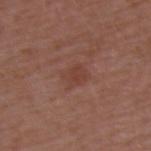Captured during whole-body skin photography for melanoma surveillance; the lesion was not biopsied.
The subject is a male aged approximately 50.
A roughly 15 mm field-of-view crop from a total-body skin photograph.
This is a white-light tile.
Located on the back.
The lesion-visualizer software estimated a footprint of about 6 mm², an outline eccentricity of about 0.7 (0 = round, 1 = elongated), and two-axis asymmetry of about 0.25. The analysis additionally found a mean CIELAB color near L≈41 a*≈22 b*≈26, a lesion–skin lightness drop of about 5, and a normalized lesion–skin contrast near 5. And it measured a border-irregularity index near 3/10, a color-variation rating of about 2/10, and radial color variation of about 0.5. The analysis additionally found a lesion-detection confidence of about 100/100.
Approximately 3 mm at its widest.